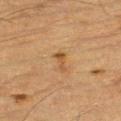Q: Was a biopsy performed?
A: catalogued during a skin exam; not biopsied
Q: What lighting was used for the tile?
A: cross-polarized
Q: What is the lesion's diameter?
A: about 2.5 mm
Q: What are the patient's age and sex?
A: male, aged approximately 85 or older
Q: What did automated image analysis measure?
A: a mean CIELAB color near L≈45 a*≈18 b*≈36 and a lesion–skin lightness drop of about 8; a classifier nevus-likeness of about 5/100 and a detector confidence of about 100 out of 100 that the crop contains a lesion
Q: Lesion location?
A: the left thigh
Q: How was this image acquired?
A: total-body-photography crop, ~15 mm field of view